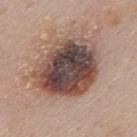Assessment:
Recorded during total-body skin imaging; not selected for excision or biopsy.
Background:
An algorithmic analysis of the crop reported an area of roughly 38 mm². The software also gave a classifier nevus-likeness of about 5/100. The recorded lesion diameter is about 7.5 mm. The subject is a male about 50 years old. This is a white-light tile. A 15 mm close-up tile from a total-body photography series done for melanoma screening. On the mid back.